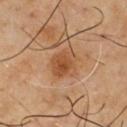Impression: Recorded during total-body skin imaging; not selected for excision or biopsy. Background: The lesion's longest dimension is about 4.5 mm. A male subject, about 50 years old. A roughly 15 mm field-of-view crop from a total-body skin photograph. From the chest.